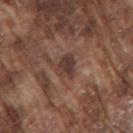Captured during whole-body skin photography for melanoma surveillance; the lesion was not biopsied. A roughly 15 mm field-of-view crop from a total-body skin photograph. A male patient, aged approximately 75. The lesion is located on the right upper arm. Captured under white-light illumination. About 3 mm across.